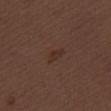Notes:
- subject · male, in their 70s
- illumination · white-light
- automated lesion analysis · an area of roughly 2.5 mm² and two-axis asymmetry of about 0.3; border irregularity of about 3 on a 0–10 scale, a color-variation rating of about 0.5/10, and a peripheral color-asymmetry measure near 0
- image · 15 mm crop, total-body photography
- lesion diameter · ≈2.5 mm
- anatomic site · the right thigh